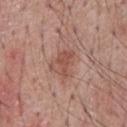Case summary:
* notes · total-body-photography surveillance lesion; no biopsy
* lesion size · about 3.5 mm
* TBP lesion metrics · a shape eccentricity near 0.75 and two-axis asymmetry of about 0.35; a border-irregularity index near 4/10 and a color-variation rating of about 4/10; a lesion-detection confidence of about 100/100
* illumination · white-light illumination
* location · the front of the torso
* patient · male, aged 63 to 67
* imaging modality · ~15 mm crop, total-body skin-cancer survey